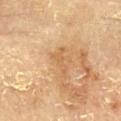Recorded during total-body skin imaging; not selected for excision or biopsy. Automated image analysis of the tile measured a lesion color around L≈60 a*≈20 b*≈39 in CIELAB and a normalized lesion–skin contrast near 5. The analysis additionally found a border-irregularity rating of about 7/10, a within-lesion color-variation index near 0/10, and peripheral color asymmetry of about 0. Imaged with cross-polarized lighting. A region of skin cropped from a whole-body photographic capture, roughly 15 mm wide. A male subject in their mid-80s or older. Approximately 3 mm at its widest. The lesion is on the chest.